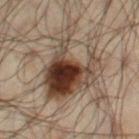{
  "patient": {
    "sex": "male",
    "age_approx": 50
  },
  "lighting": "cross-polarized",
  "image": {
    "source": "total-body photography crop",
    "field_of_view_mm": 15
  },
  "site": "right thigh"
}